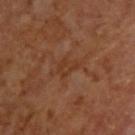Findings:
• biopsy status · imaged on a skin check; not biopsied
• tile lighting · cross-polarized
• body site · the upper back
• TBP lesion metrics · a shape eccentricity near 0.7 and a symmetry-axis asymmetry near 0.45; an average lesion color of about L≈35 a*≈21 b*≈31 (CIELAB) and a normalized lesion–skin contrast near 5.5; a classifier nevus-likeness of about 0/100 and a lesion-detection confidence of about 95/100
• patient · male, aged 63 to 67
• image source · ~15 mm crop, total-body skin-cancer survey